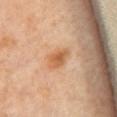workup: total-body-photography surveillance lesion; no biopsy
diameter: ≈3.5 mm
image: total-body-photography crop, ~15 mm field of view
patient: female, in their mid-60s
anatomic site: the front of the torso
illumination: cross-polarized illumination